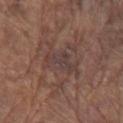Findings:
* biopsy status: total-body-photography surveillance lesion; no biopsy
* image: ~15 mm tile from a whole-body skin photo
* tile lighting: white-light
* subject: male, approximately 80 years of age
* size: ≈4 mm
* site: the arm
* TBP lesion metrics: an area of roughly 6 mm², an eccentricity of roughly 0.8, and two-axis asymmetry of about 0.5; a peripheral color-asymmetry measure near 0.5; a nevus-likeness score of about 0/100 and a detector confidence of about 50 out of 100 that the crop contains a lesion